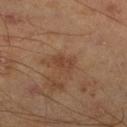Q: Is there a histopathology result?
A: imaged on a skin check; not biopsied
Q: What is the lesion's diameter?
A: ≈2.5 mm
Q: Patient demographics?
A: male, aged 43 to 47
Q: How was the tile lit?
A: cross-polarized
Q: What is the imaging modality?
A: total-body-photography crop, ~15 mm field of view
Q: Automated lesion metrics?
A: border irregularity of about 1.5 on a 0–10 scale, a color-variation rating of about 2.5/10, and radial color variation of about 1
Q: Lesion location?
A: the right lower leg